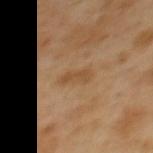The lesion was tiled from a total-body skin photograph and was not biopsied. A region of skin cropped from a whole-body photographic capture, roughly 15 mm wide. A male subject, aged 48–52. Located on the back. Automated tile analysis of the lesion measured border irregularity of about 4 on a 0–10 scale and radial color variation of about 0.5. And it measured an automated nevus-likeness rating near 0 out of 100 and a detector confidence of about 100 out of 100 that the crop contains a lesion. This is a cross-polarized tile. Approximately 3.5 mm at its widest.